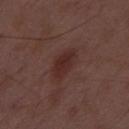follow-up: imaged on a skin check; not biopsied
lighting: white-light illumination
lesion size: ~4 mm (longest diameter)
automated metrics: an area of roughly 7 mm² and two-axis asymmetry of about 0.2; a mean CIELAB color near L≈28 a*≈20 b*≈20, roughly 7 lightness units darker than nearby skin, and a lesion-to-skin contrast of about 7.5 (normalized; higher = more distinct); a detector confidence of about 100 out of 100 that the crop contains a lesion
acquisition: ~15 mm crop, total-body skin-cancer survey
subject: male, approximately 50 years of age